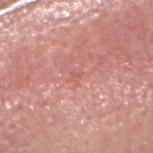No biopsy was performed on this lesion — it was imaged during a full skin examination and was not determined to be concerning.
Approximately 1.5 mm at its widest.
The patient is a male aged approximately 60.
A close-up tile cropped from a whole-body skin photograph, about 15 mm across.
The lesion is located on the head or neck.
Imaged with white-light lighting.